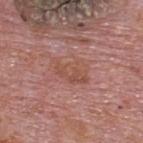Background: On the upper back. Imaged with white-light lighting. Automated image analysis of the tile measured an area of roughly 9 mm², an outline eccentricity of about 0.8 (0 = round, 1 = elongated), and a shape-asymmetry score of about 0.35 (0 = symmetric). The software also gave border irregularity of about 4 on a 0–10 scale, internal color variation of about 3.5 on a 0–10 scale, and radial color variation of about 1.5. This image is a 15 mm lesion crop taken from a total-body photograph. A male patient, roughly 75 years of age.